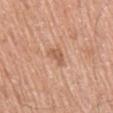biopsy status: no biopsy performed (imaged during a skin exam); image: ~15 mm crop, total-body skin-cancer survey; body site: the right upper arm; subject: male, aged 58–62.